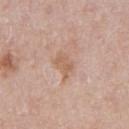<lesion>
<biopsy_status>not biopsied; imaged during a skin examination</biopsy_status>
<lesion_size>
  <long_diameter_mm_approx>3.0</long_diameter_mm_approx>
</lesion_size>
<patient>
  <sex>male</sex>
  <age_approx>60</age_approx>
</patient>
<site>front of the torso</site>
<automated_metrics>
  <area_mm2_approx>5.0</area_mm2_approx>
  <shape_asymmetry>0.3</shape_asymmetry>
  <border_irregularity_0_10>3.5</border_irregularity_0_10>
  <color_variation_0_10>2.0</color_variation_0_10>
  <nevus_likeness_0_100>0</nevus_likeness_0_100>
  <lesion_detection_confidence_0_100>100</lesion_detection_confidence_0_100>
</automated_metrics>
<lighting>white-light</lighting>
<image>
  <source>total-body photography crop</source>
  <field_of_view_mm>15</field_of_view_mm>
</image>
</lesion>